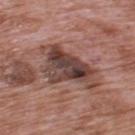<lesion>
  <biopsy_status>not biopsied; imaged during a skin examination</biopsy_status>
  <patient>
    <sex>male</sex>
    <age_approx>70</age_approx>
  </patient>
  <lesion_size>
    <long_diameter_mm_approx>7.5</long_diameter_mm_approx>
  </lesion_size>
  <site>upper back</site>
  <automated_metrics>
    <border_irregularity_0_10>5.5</border_irregularity_0_10>
    <color_variation_0_10>9.0</color_variation_0_10>
    <peripheral_color_asymmetry>3.0</peripheral_color_asymmetry>
  </automated_metrics>
  <image>
    <source>total-body photography crop</source>
    <field_of_view_mm>15</field_of_view_mm>
  </image>
  <lighting>white-light</lighting>
</lesion>